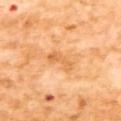Q: Was a biopsy performed?
A: no biopsy performed (imaged during a skin exam)
Q: What are the patient's age and sex?
A: female, about 55 years old
Q: How was this image acquired?
A: 15 mm crop, total-body photography
Q: How was the tile lit?
A: cross-polarized
Q: Lesion size?
A: about 3 mm
Q: Automated lesion metrics?
A: a footprint of about 4.5 mm², an outline eccentricity of about 0.85 (0 = round, 1 = elongated), and two-axis asymmetry of about 0.45; a classifier nevus-likeness of about 0/100 and lesion-presence confidence of about 100/100
Q: What is the anatomic site?
A: the upper back